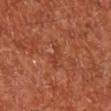Findings:
• biopsy status — catalogued during a skin exam; not biopsied
• anatomic site — the leg
• imaging modality — total-body-photography crop, ~15 mm field of view
• patient — male, about 65 years old